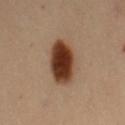body site = the mid back; image = 15 mm crop, total-body photography; size = about 6 mm; subject = female, in their 50s; illumination = cross-polarized.